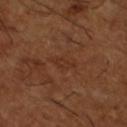<tbp_lesion>
<biopsy_status>not biopsied; imaged during a skin examination</biopsy_status>
<lighting>cross-polarized</lighting>
<patient>
  <sex>male</sex>
  <age_approx>65</age_approx>
</patient>
<site>left lower leg</site>
<image>
  <source>total-body photography crop</source>
  <field_of_view_mm>15</field_of_view_mm>
</image>
<lesion_size>
  <long_diameter_mm_approx>3.0</long_diameter_mm_approx>
</lesion_size>
</tbp_lesion>